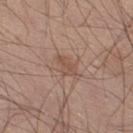{
  "biopsy_status": "not biopsied; imaged during a skin examination",
  "image": {
    "source": "total-body photography crop",
    "field_of_view_mm": 15
  },
  "site": "right lower leg",
  "lesion_size": {
    "long_diameter_mm_approx": 3.0
  },
  "lighting": "white-light",
  "patient": {
    "sex": "male",
    "age_approx": 45
  },
  "automated_metrics": {
    "vs_skin_darker_L": 7.0,
    "vs_skin_contrast_norm": 5.5,
    "border_irregularity_0_10": 3.0,
    "color_variation_0_10": 1.5,
    "nevus_likeness_0_100": 0,
    "lesion_detection_confidence_0_100": 100
  }
}